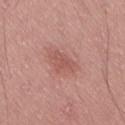| field | value |
|---|---|
| notes | total-body-photography surveillance lesion; no biopsy |
| subject | male, aged 48–52 |
| imaging modality | ~15 mm tile from a whole-body skin photo |
| diameter | about 4 mm |
| illumination | white-light illumination |
| anatomic site | the lower back |